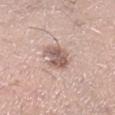| key | value |
|---|---|
| site | the right lower leg |
| acquisition | total-body-photography crop, ~15 mm field of view |
| patient | male, about 35 years old |